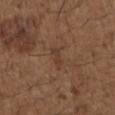biopsy status: total-body-photography surveillance lesion; no biopsy | illumination: white-light | body site: the left upper arm | TBP lesion metrics: peripheral color asymmetry of about 0 | lesion diameter: ~2.5 mm (longest diameter) | image source: ~15 mm crop, total-body skin-cancer survey | patient: male, in their 50s.